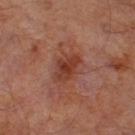Clinical impression:
Recorded during total-body skin imaging; not selected for excision or biopsy.
Image and clinical context:
A 15 mm close-up tile from a total-body photography series done for melanoma screening. The recorded lesion diameter is about 4 mm. A male patient, in their mid-60s. From the right thigh. Imaged with cross-polarized lighting.